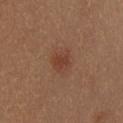Acquisition and patient details:
The patient is a female in their mid-20s. From the head or neck. A 15 mm crop from a total-body photograph taken for skin-cancer surveillance.